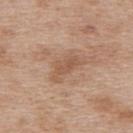Acquisition and patient details: This image is a 15 mm lesion crop taken from a total-body photograph. From the back. A female patient aged 38–42.